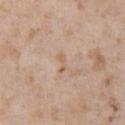Q: Lesion location?
A: the right upper arm
Q: How large is the lesion?
A: ≈2.5 mm
Q: What did automated image analysis measure?
A: an area of roughly 2 mm² and a symmetry-axis asymmetry near 0.6; an average lesion color of about L≈61 a*≈17 b*≈31 (CIELAB), a lesion–skin lightness drop of about 7, and a lesion-to-skin contrast of about 5.5 (normalized; higher = more distinct); peripheral color asymmetry of about 0
Q: What lighting was used for the tile?
A: white-light
Q: What is the imaging modality?
A: 15 mm crop, total-body photography
Q: What are the patient's age and sex?
A: male, aged approximately 60